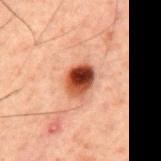Impression:
Captured during whole-body skin photography for melanoma surveillance; the lesion was not biopsied.